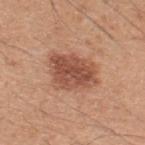Q: Is there a histopathology result?
A: total-body-photography surveillance lesion; no biopsy
Q: What are the patient's age and sex?
A: male, aged approximately 45
Q: What kind of image is this?
A: 15 mm crop, total-body photography
Q: Lesion size?
A: ~5.5 mm (longest diameter)
Q: What lighting was used for the tile?
A: white-light illumination
Q: Lesion location?
A: the upper back